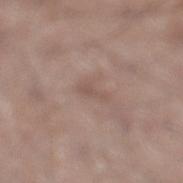Case summary:
- automated metrics — a footprint of about 2 mm², an eccentricity of roughly 0.95, and a symmetry-axis asymmetry near 0.5; a border-irregularity rating of about 6/10 and a within-lesion color-variation index near 0/10
- lesion diameter — about 3 mm
- lighting — white-light
- image — ~15 mm crop, total-body skin-cancer survey
- anatomic site — the left lower leg
- patient — male, in their 60s